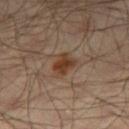| field | value |
|---|---|
| follow-up | no biopsy performed (imaged during a skin exam) |
| subject | male, in their mid- to late 60s |
| site | the right thigh |
| illumination | cross-polarized illumination |
| imaging modality | ~15 mm tile from a whole-body skin photo |